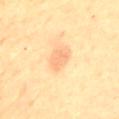{
  "site": "mid back",
  "image": {
    "source": "total-body photography crop",
    "field_of_view_mm": 15
  },
  "lighting": "cross-polarized",
  "lesion_size": {
    "long_diameter_mm_approx": 2.5
  },
  "patient": {
    "sex": "female",
    "age_approx": 80
  },
  "automated_metrics": {
    "area_mm2_approx": 3.5,
    "eccentricity": 0.8,
    "shape_asymmetry": 0.3,
    "cielab_L": 66,
    "cielab_a": 20,
    "cielab_b": 37,
    "vs_skin_contrast_norm": 5.0,
    "border_irregularity_0_10": 3.0,
    "peripheral_color_asymmetry": 0.5
  }
}